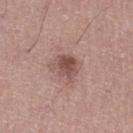  biopsy_status: not biopsied; imaged during a skin examination
  patient:
    sex: male
    age_approx: 45
  lesion_size:
    long_diameter_mm_approx: 3.0
  site: left lower leg
  image:
    source: total-body photography crop
    field_of_view_mm: 15
  lighting: white-light